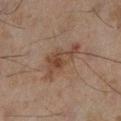notes: total-body-photography surveillance lesion; no biopsy | anatomic site: the left lower leg | image source: ~15 mm crop, total-body skin-cancer survey | automated lesion analysis: an outline eccentricity of about 0.9 (0 = round, 1 = elongated) and two-axis asymmetry of about 0.5; a nevus-likeness score of about 5/100 and a lesion-detection confidence of about 100/100 | subject: male, aged approximately 45 | lesion diameter: ≈5.5 mm | illumination: cross-polarized illumination.